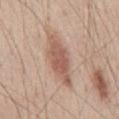biopsy status = no biopsy performed (imaged during a skin exam)
illumination = white-light illumination
patient = male, aged approximately 45
automated metrics = an area of roughly 13 mm² and an eccentricity of roughly 0.95; about 11 CIELAB-L* units darker than the surrounding skin
body site = the chest
imaging modality = 15 mm crop, total-body photography
diameter = ≈7 mm